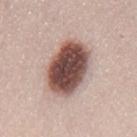biopsy_status: not biopsied; imaged during a skin examination
patient:
  sex: female
  age_approx: 50
lesion_size:
  long_diameter_mm_approx: 6.5
automated_metrics:
  area_mm2_approx: 24.0
  eccentricity: 0.7
  shape_asymmetry: 0.1
  cielab_L: 49
  cielab_a: 19
  cielab_b: 22
  vs_skin_darker_L: 23.0
  vs_skin_contrast_norm: 14.5
  border_irregularity_0_10: 1.5
  color_variation_0_10: 6.5
  peripheral_color_asymmetry: 2.0
  nevus_likeness_0_100: 85
  lesion_detection_confidence_0_100: 100
image:
  source: total-body photography crop
  field_of_view_mm: 15
site: mid back